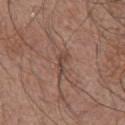Clinical impression:
Imaged during a routine full-body skin examination; the lesion was not biopsied and no histopathology is available.
Background:
A 15 mm close-up tile from a total-body photography series done for melanoma screening. A male subject, roughly 65 years of age. The lesion's longest dimension is about 3 mm. This is a white-light tile. The lesion is located on the chest. Automated image analysis of the tile measured a lesion area of about 3.5 mm² and a shape-asymmetry score of about 0.35 (0 = symmetric). It also reported a mean CIELAB color near L≈45 a*≈18 b*≈24, a lesion–skin lightness drop of about 8, and a lesion-to-skin contrast of about 6.5 (normalized; higher = more distinct).